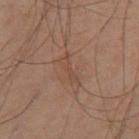<tbp_lesion>
<biopsy_status>not biopsied; imaged during a skin examination</biopsy_status>
<patient>
  <sex>male</sex>
  <age_approx>60</age_approx>
</patient>
<image>
  <source>total-body photography crop</source>
  <field_of_view_mm>15</field_of_view_mm>
</image>
<site>left thigh</site>
</tbp_lesion>